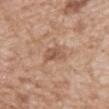workup = catalogued during a skin exam; not biopsied
location = the chest
patient = male, approximately 60 years of age
tile lighting = white-light
acquisition = 15 mm crop, total-body photography